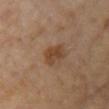biopsy status: imaged on a skin check; not biopsied.